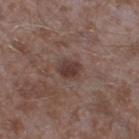notes: no biopsy performed (imaged during a skin exam); image source: ~15 mm tile from a whole-body skin photo; diameter: ~2.5 mm (longest diameter); patient: male, approximately 45 years of age; illumination: white-light; site: the leg.